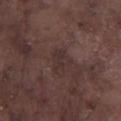About 3 mm across. A male subject in their mid- to late 70s. A roughly 15 mm field-of-view crop from a total-body skin photograph. The tile uses white-light illumination. The lesion is located on the left lower leg. The total-body-photography lesion software estimated roughly 5 lightness units darker than nearby skin and a lesion-to-skin contrast of about 5.5 (normalized; higher = more distinct). The analysis additionally found a color-variation rating of about 1.5/10 and a peripheral color-asymmetry measure near 0.5.